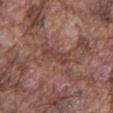Findings:
– image-analysis metrics: border irregularity of about 5.5 on a 0–10 scale, internal color variation of about 1 on a 0–10 scale, and a peripheral color-asymmetry measure near 0.5
– diameter: ~3.5 mm (longest diameter)
– body site: the mid back
– lighting: white-light illumination
– subject: male, aged around 75
– image: total-body-photography crop, ~15 mm field of view